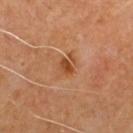Part of a total-body skin-imaging series; this lesion was reviewed on a skin check and was not flagged for biopsy. A region of skin cropped from a whole-body photographic capture, roughly 15 mm wide. The lesion is located on the chest. A male subject about 60 years old.